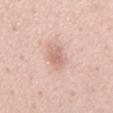{"biopsy_status": "not biopsied; imaged during a skin examination", "image": {"source": "total-body photography crop", "field_of_view_mm": 15}, "lesion_size": {"long_diameter_mm_approx": 4.0}, "patient": {"sex": "male", "age_approx": 55}, "lighting": "white-light", "site": "chest"}